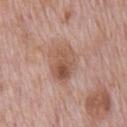Impression:
No biopsy was performed on this lesion — it was imaged during a full skin examination and was not determined to be concerning.
Acquisition and patient details:
The lesion's longest dimension is about 5 mm. A lesion tile, about 15 mm wide, cut from a 3D total-body photograph. The lesion is on the chest. The subject is a male aged approximately 70.